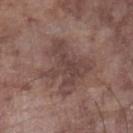Background:
Longest diameter approximately 6 mm. The lesion is located on the left lower leg. An algorithmic analysis of the crop reported roughly 8 lightness units darker than nearby skin and a normalized lesion–skin contrast near 6.5. A male patient, in their mid- to late 70s. Cropped from a total-body skin-imaging series; the visible field is about 15 mm.